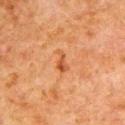Background: A 15 mm close-up extracted from a 3D total-body photography capture. This is a cross-polarized tile. The recorded lesion diameter is about 3 mm. A male subject, approximately 80 years of age. Located on the left upper arm.